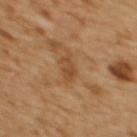biopsy_status: not biopsied; imaged during a skin examination
site: upper back
image:
  source: total-body photography crop
  field_of_view_mm: 15
patient:
  sex: female
  age_approx: 55
automated_metrics:
  cielab_L: 48
  cielab_a: 20
  cielab_b: 37
  vs_skin_contrast_norm: 6.0